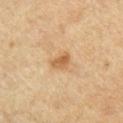imaging modality — total-body-photography crop, ~15 mm field of view
tile lighting — cross-polarized
anatomic site — the arm
subject — male, about 55 years old
TBP lesion metrics — an average lesion color of about L≈59 a*≈19 b*≈39 (CIELAB), roughly 10 lightness units darker than nearby skin, and a normalized border contrast of about 7.5; a color-variation rating of about 3.5/10 and peripheral color asymmetry of about 1